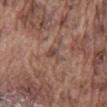Background:
Cropped from a whole-body photographic skin survey; the tile spans about 15 mm. On the mid back. The subject is a male aged approximately 75. This is a white-light tile. Measured at roughly 2.5 mm in maximum diameter.